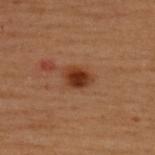Captured during whole-body skin photography for melanoma surveillance; the lesion was not biopsied.
Captured under cross-polarized illumination.
The recorded lesion diameter is about 3.5 mm.
The patient is a female aged approximately 50.
The lesion is on the upper back.
This image is a 15 mm lesion crop taken from a total-body photograph.